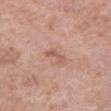No biopsy was performed on this lesion — it was imaged during a full skin examination and was not determined to be concerning. Measured at roughly 2.5 mm in maximum diameter. A male patient, approximately 70 years of age. On the chest. Cropped from a total-body skin-imaging series; the visible field is about 15 mm.